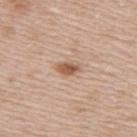patient — female, in their 50s | location — the right upper arm | TBP lesion metrics — an automated nevus-likeness rating near 95 out of 100 and a lesion-detection confidence of about 100/100 | imaging modality — total-body-photography crop, ~15 mm field of view | lesion diameter — ~2.5 mm (longest diameter) | illumination — white-light illumination.